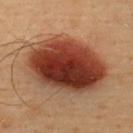biopsy status — imaged on a skin check; not biopsied
TBP lesion metrics — a symmetry-axis asymmetry near 0.1; a lesion color around L≈40 a*≈27 b*≈31 in CIELAB and a normalized lesion–skin contrast near 14.5
subject — male, aged 38 to 42
body site — the upper back
imaging modality — ~15 mm crop, total-body skin-cancer survey
size — ≈9.5 mm
lighting — cross-polarized illumination